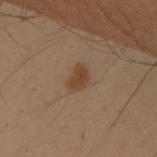biopsy status: imaged on a skin check; not biopsied
subject: female, aged approximately 50
anatomic site: the left upper arm
automated lesion analysis: a lesion–skin lightness drop of about 7 and a normalized border contrast of about 8; a border-irregularity index near 2.5/10, internal color variation of about 1.5 on a 0–10 scale, and radial color variation of about 0.5; an automated nevus-likeness rating near 95 out of 100 and a lesion-detection confidence of about 100/100
acquisition: ~15 mm crop, total-body skin-cancer survey